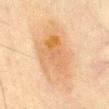lighting: cross-polarized
patient:
  sex: male
  age_approx: 75
site: chest
lesion_size:
  long_diameter_mm_approx: 9.5
image:
  source: total-body photography crop
  field_of_view_mm: 15